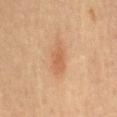image-analysis metrics: an area of roughly 7.5 mm², an outline eccentricity of about 0.9 (0 = round, 1 = elongated), and a symmetry-axis asymmetry near 0.2; a nevus-likeness score of about 65/100 and lesion-presence confidence of about 100/100 | subject: female, aged 63–67 | acquisition: ~15 mm tile from a whole-body skin photo | body site: the mid back | lighting: cross-polarized | lesion size: ~5 mm (longest diameter).